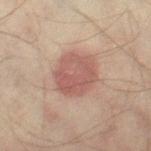Recorded during total-body skin imaging; not selected for excision or biopsy.
A close-up tile cropped from a whole-body skin photograph, about 15 mm across.
Measured at roughly 4.5 mm in maximum diameter.
The tile uses cross-polarized illumination.
The subject is a male aged around 45.
The lesion is located on the right thigh.